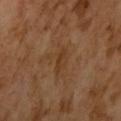workup=imaged on a skin check; not biopsied | patient=male, about 65 years old | image source=~15 mm crop, total-body skin-cancer survey.